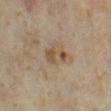The lesion was tiled from a total-body skin photograph and was not biopsied.
The lesion is on the left thigh.
A 15 mm crop from a total-body photograph taken for skin-cancer surveillance.
An algorithmic analysis of the crop reported a lesion area of about 4.5 mm² and an eccentricity of roughly 0.85. And it measured a normalized border contrast of about 7.5.
Imaged with cross-polarized lighting.
The lesion's longest dimension is about 3.5 mm.
A female subject, aged approximately 35.